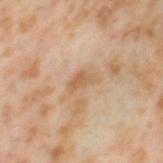Q: Was this lesion biopsied?
A: total-body-photography surveillance lesion; no biopsy
Q: How was this image acquired?
A: total-body-photography crop, ~15 mm field of view
Q: How large is the lesion?
A: ~3 mm (longest diameter)
Q: Automated lesion metrics?
A: a footprint of about 4 mm², an eccentricity of roughly 0.8, and a symmetry-axis asymmetry near 0.45
Q: Patient demographics?
A: female, aged approximately 55
Q: Where on the body is the lesion?
A: the left thigh
Q: Illumination type?
A: cross-polarized